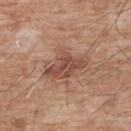Notes:
– workup — no biopsy performed (imaged during a skin exam)
– size — ~5.5 mm (longest diameter)
– automated lesion analysis — a lesion area of about 12 mm², an outline eccentricity of about 0.8 (0 = round, 1 = elongated), and two-axis asymmetry of about 0.35; a lesion color around L≈49 a*≈21 b*≈28 in CIELAB, about 11 CIELAB-L* units darker than the surrounding skin, and a normalized border contrast of about 7.5; a border-irregularity index near 4.5/10 and a color-variation rating of about 3.5/10; lesion-presence confidence of about 100/100
– lighting — white-light illumination
– imaging modality — total-body-photography crop, ~15 mm field of view
– anatomic site — the chest
– subject — male, roughly 60 years of age